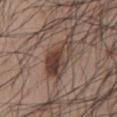biopsy_status: not biopsied; imaged during a skin examination
site: mid back
patient:
  sex: male
  age_approx: 70
image:
  source: total-body photography crop
  field_of_view_mm: 15
lesion_size:
  long_diameter_mm_approx: 6.0
automated_metrics:
  border_irregularity_0_10: 4.5
  color_variation_0_10: 9.0
  peripheral_color_asymmetry: 4.0